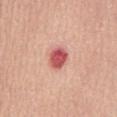Assessment:
Captured during whole-body skin photography for melanoma surveillance; the lesion was not biopsied.
Clinical summary:
A roughly 15 mm field-of-view crop from a total-body skin photograph. Located on the abdomen. About 3 mm across. Automated image analysis of the tile measured an area of roughly 6 mm², an outline eccentricity of about 0.6 (0 = round, 1 = elongated), and a symmetry-axis asymmetry near 0.2. The software also gave a mean CIELAB color near L≈57 a*≈34 b*≈26, a lesion–skin lightness drop of about 16, and a lesion-to-skin contrast of about 10 (normalized; higher = more distinct). It also reported a border-irregularity rating of about 1.5/10, a within-lesion color-variation index near 4.5/10, and radial color variation of about 1.5. A male subject, in their mid-60s. Imaged with white-light lighting.